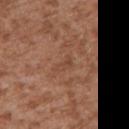Acquisition and patient details:
The patient is a male aged 43–47. The recorded lesion diameter is about 2.5 mm. Automated image analysis of the tile measured a border-irregularity rating of about 5/10, a within-lesion color-variation index near 0/10, and peripheral color asymmetry of about 0. The software also gave a classifier nevus-likeness of about 0/100 and a lesion-detection confidence of about 100/100. A lesion tile, about 15 mm wide, cut from a 3D total-body photograph. The lesion is on the left upper arm.